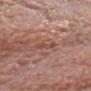This lesion was catalogued during total-body skin photography and was not selected for biopsy. The lesion's longest dimension is about 3.5 mm. Imaged with white-light lighting. A roughly 15 mm field-of-view crop from a total-body skin photograph. Automated tile analysis of the lesion measured an average lesion color of about L≈48 a*≈24 b*≈26 (CIELAB), roughly 7 lightness units darker than nearby skin, and a lesion-to-skin contrast of about 5.5 (normalized; higher = more distinct). The software also gave a border-irregularity index near 6/10, a color-variation rating of about 0/10, and radial color variation of about 0. The analysis additionally found a nevus-likeness score of about 0/100 and a lesion-detection confidence of about 65/100. A male patient approximately 65 years of age. The lesion is located on the head or neck.